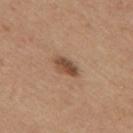biopsy status=no biopsy performed (imaged during a skin exam)
anatomic site=the mid back
subject=female, in their mid- to late 40s
tile lighting=white-light illumination
lesion size=≈3.5 mm
image source=total-body-photography crop, ~15 mm field of view